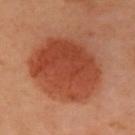Case summary:
• follow-up — catalogued during a skin exam; not biopsied